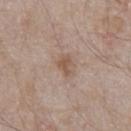Q: Is there a histopathology result?
A: imaged on a skin check; not biopsied
Q: Where on the body is the lesion?
A: the abdomen
Q: What kind of image is this?
A: ~15 mm crop, total-body skin-cancer survey
Q: What are the patient's age and sex?
A: male, aged 63 to 67